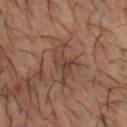notes = catalogued during a skin exam; not biopsied | image source = ~15 mm tile from a whole-body skin photo | subject = male, aged around 50 | site = the head or neck.